Measured at roughly 1.5 mm in maximum diameter · on the right thigh · Automated image analysis of the tile measured a footprint of about 1 mm², an outline eccentricity of about 0.5 (0 = round, 1 = elongated), and a shape-asymmetry score of about 0.3 (0 = symmetric). The analysis additionally found a nevus-likeness score of about 0/100 and a lesion-detection confidence of about 100/100 · a male subject, aged 43 to 47 · this is a white-light tile · this image is a 15 mm lesion crop taken from a total-body photograph:
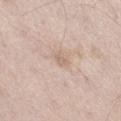The biopsy diagnosis was a solar lentigo — a benign lesion.